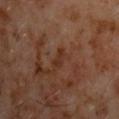• biopsy status: imaged on a skin check; not biopsied
• image: 15 mm crop, total-body photography
• subject: male, about 60 years old
• body site: the chest
• lesion size: ≈2.5 mm
• automated lesion analysis: a mean CIELAB color near L≈29 a*≈20 b*≈26 and a lesion-to-skin contrast of about 6 (normalized; higher = more distinct)
• illumination: cross-polarized